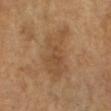<lesion>
  <biopsy_status>not biopsied; imaged during a skin examination</biopsy_status>
  <lesion_size>
    <long_diameter_mm_approx>7.0</long_diameter_mm_approx>
  </lesion_size>
  <site>right lower leg</site>
  <automated_metrics>
    <area_mm2_approx>17.0</area_mm2_approx>
    <eccentricity>0.85</eccentricity>
    <shape_asymmetry>0.4</shape_asymmetry>
    <nevus_likeness_0_100>0</nevus_likeness_0_100>
    <lesion_detection_confidence_0_100>100</lesion_detection_confidence_0_100>
  </automated_metrics>
  <patient>
    <sex>female</sex>
    <age_approx>55</age_approx>
  </patient>
  <lighting>cross-polarized</lighting>
  <image>
    <source>total-body photography crop</source>
    <field_of_view_mm>15</field_of_view_mm>
  </image>
</lesion>